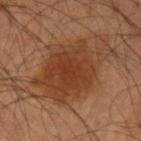Clinical summary: A 15 mm crop from a total-body photograph taken for skin-cancer surveillance. A male subject, aged 58–62. The lesion is on the right upper arm. The tile uses cross-polarized illumination. The lesion-visualizer software estimated an average lesion color of about L≈35 a*≈20 b*≈30 (CIELAB), about 8 CIELAB-L* units darker than the surrounding skin, and a normalized lesion–skin contrast near 8. About 8 mm across.